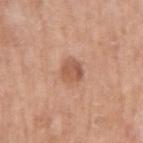Captured during whole-body skin photography for melanoma surveillance; the lesion was not biopsied. A male patient, roughly 65 years of age. A 15 mm close-up extracted from a 3D total-body photography capture. The lesion's longest dimension is about 2.5 mm. Automated image analysis of the tile measured a footprint of about 6 mm², an eccentricity of roughly 0.45, and a symmetry-axis asymmetry near 0.2. Imaged with white-light lighting. The lesion is located on the arm.